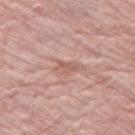tile lighting = white-light illumination
image = ~15 mm crop, total-body skin-cancer survey
body site = the leg
size = about 3 mm
subject = female, approximately 70 years of age